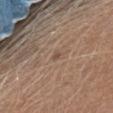Part of a total-body skin-imaging series; this lesion was reviewed on a skin check and was not flagged for biopsy. The patient is a female aged approximately 55. Measured at roughly 1 mm in maximum diameter. This is a white-light tile. A roughly 15 mm field-of-view crop from a total-body skin photograph. On the head or neck.